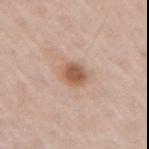The lesion was tiled from a total-body skin photograph and was not biopsied. The lesion is located on the right upper arm. A region of skin cropped from a whole-body photographic capture, roughly 15 mm wide. Measured at roughly 3 mm in maximum diameter. Imaged with white-light lighting. The patient is a female aged approximately 50.